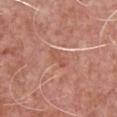Part of a total-body skin-imaging series; this lesion was reviewed on a skin check and was not flagged for biopsy. Approximately 3 mm at its widest. Imaged with white-light lighting. Located on the chest. A region of skin cropped from a whole-body photographic capture, roughly 15 mm wide. The subject is a male in their mid- to late 50s.